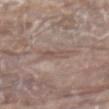Recorded during total-body skin imaging; not selected for excision or biopsy.
An algorithmic analysis of the crop reported about 6 CIELAB-L* units darker than the surrounding skin and a normalized lesion–skin contrast near 4.5. It also reported peripheral color asymmetry of about 1.
A 15 mm crop from a total-body photograph taken for skin-cancer surveillance.
A male subject, approximately 80 years of age.
Captured under white-light illumination.
The lesion is located on the mid back.